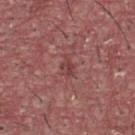Background:
Imaged with white-light lighting. About 2.5 mm across. On the front of the torso. A region of skin cropped from a whole-body photographic capture, roughly 15 mm wide. The subject is a male aged 38 to 42.